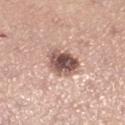<tbp_lesion>
  <biopsy_status>not biopsied; imaged during a skin examination</biopsy_status>
  <lesion_size>
    <long_diameter_mm_approx>4.5</long_diameter_mm_approx>
  </lesion_size>
  <site>left lower leg</site>
  <image>
    <source>total-body photography crop</source>
    <field_of_view_mm>15</field_of_view_mm>
  </image>
  <patient>
    <sex>female</sex>
    <age_approx>65</age_approx>
  </patient>
  <lighting>white-light</lighting>
</tbp_lesion>